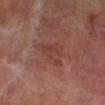{"patient": {"sex": "male", "age_approx": 70}, "image": {"source": "total-body photography crop", "field_of_view_mm": 15}, "site": "right lower leg", "lesion_size": {"long_diameter_mm_approx": 4.5}, "automated_metrics": {"eccentricity": 0.75, "shape_asymmetry": 0.45, "cielab_L": 39, "cielab_a": 23, "cielab_b": 25, "vs_skin_darker_L": 5.0, "vs_skin_contrast_norm": 5.0, "border_irregularity_0_10": 4.5, "color_variation_0_10": 2.5, "peripheral_color_asymmetry": 1.0, "nevus_likeness_0_100": 0, "lesion_detection_confidence_0_100": 100}}